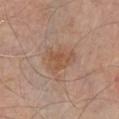| key | value |
|---|---|
| diameter | about 4 mm |
| patient | male, aged 78–82 |
| illumination | white-light |
| acquisition | ~15 mm crop, total-body skin-cancer survey |
| automated metrics | a mean CIELAB color near L≈53 a*≈19 b*≈31, roughly 7 lightness units darker than nearby skin, and a normalized border contrast of about 6; border irregularity of about 4 on a 0–10 scale, a color-variation rating of about 3/10, and radial color variation of about 1 |
| site | the left upper arm |